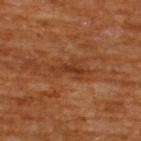follow-up: total-body-photography surveillance lesion; no biopsy | image source: total-body-photography crop, ~15 mm field of view | location: the back | subject: male, in their mid- to late 60s.